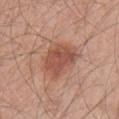– biopsy status · total-body-photography surveillance lesion; no biopsy
– diameter · ~5 mm (longest diameter)
– subject · male, approximately 45 years of age
– anatomic site · the left upper arm
– lighting · white-light
– imaging modality · ~15 mm tile from a whole-body skin photo